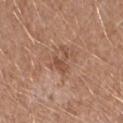Q: Was this lesion biopsied?
A: catalogued during a skin exam; not biopsied
Q: How large is the lesion?
A: about 3.5 mm
Q: Automated lesion metrics?
A: a footprint of about 4 mm², a shape eccentricity near 0.8, and a symmetry-axis asymmetry near 0.65; about 8 CIELAB-L* units darker than the surrounding skin and a lesion-to-skin contrast of about 6 (normalized; higher = more distinct); a border-irregularity index near 8/10, a within-lesion color-variation index near 1.5/10, and a peripheral color-asymmetry measure near 0.5; a classifier nevus-likeness of about 0/100
Q: Who is the patient?
A: female, aged 38–42
Q: Where on the body is the lesion?
A: the right forearm
Q: Illumination type?
A: white-light
Q: What kind of image is this?
A: 15 mm crop, total-body photography